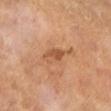Imaged during a routine full-body skin examination; the lesion was not biopsied and no histopathology is available. A male subject in their mid-60s. An algorithmic analysis of the crop reported a footprint of about 6 mm², an eccentricity of roughly 0.95, and a symmetry-axis asymmetry near 0.4. And it measured border irregularity of about 5 on a 0–10 scale, internal color variation of about 4 on a 0–10 scale, and radial color variation of about 1.5. The lesion is on the left lower leg. Measured at roughly 5 mm in maximum diameter. This is a cross-polarized tile. A region of skin cropped from a whole-body photographic capture, roughly 15 mm wide.